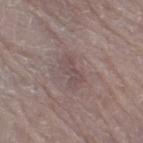follow-up=imaged on a skin check; not biopsied
subject=male, in their 80s
body site=the leg
lighting=white-light
image=total-body-photography crop, ~15 mm field of view